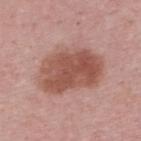Findings:
• workup — imaged on a skin check; not biopsied